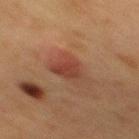Impression:
Recorded during total-body skin imaging; not selected for excision or biopsy.
Image and clinical context:
A female patient aged 38 to 42. Imaged with cross-polarized lighting. From the mid back. The lesion's longest dimension is about 4 mm. A close-up tile cropped from a whole-body skin photograph, about 15 mm across. Automated image analysis of the tile measured a footprint of about 9 mm², an outline eccentricity of about 0.75 (0 = round, 1 = elongated), and two-axis asymmetry of about 0.4. The software also gave border irregularity of about 4 on a 0–10 scale and internal color variation of about 4 on a 0–10 scale.